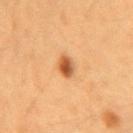Imaged during a routine full-body skin examination; the lesion was not biopsied and no histopathology is available. Cropped from a whole-body photographic skin survey; the tile spans about 15 mm. A female patient, in their 40s. From the arm.